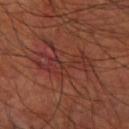Part of a total-body skin-imaging series; this lesion was reviewed on a skin check and was not flagged for biopsy.
Longest diameter approximately 7 mm.
The total-body-photography lesion software estimated an area of roughly 14 mm², an outline eccentricity of about 0.95 (0 = round, 1 = elongated), and two-axis asymmetry of about 0.55. And it measured an average lesion color of about L≈33 a*≈26 b*≈25 (CIELAB), about 6 CIELAB-L* units darker than the surrounding skin, and a normalized lesion–skin contrast near 5.5. And it measured a within-lesion color-variation index near 5/10 and a peripheral color-asymmetry measure near 1.5. The software also gave a nevus-likeness score of about 0/100.
Imaged with cross-polarized lighting.
A 15 mm crop from a total-body photograph taken for skin-cancer surveillance.
Located on the arm.
A male subject approximately 65 years of age.